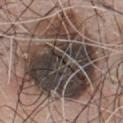{
  "biopsy_status": "not biopsied; imaged during a skin examination",
  "site": "chest",
  "lesion_size": {
    "long_diameter_mm_approx": 13.5
  },
  "automated_metrics": {
    "area_mm2_approx": 100.0,
    "eccentricity": 0.5,
    "shape_asymmetry": 0.3,
    "cielab_L": 43,
    "cielab_a": 11,
    "cielab_b": 18,
    "vs_skin_darker_L": 16.0
  },
  "image": {
    "source": "total-body photography crop",
    "field_of_view_mm": 15
  },
  "patient": {
    "sex": "male",
    "age_approx": 75
  }
}